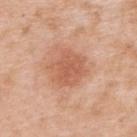workup=catalogued during a skin exam; not biopsied
image source=15 mm crop, total-body photography
patient=female, about 40 years old
site=the upper back
size=≈4.5 mm